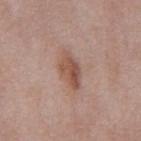Clinical impression:
This lesion was catalogued during total-body skin photography and was not selected for biopsy.
Acquisition and patient details:
Automated image analysis of the tile measured an outline eccentricity of about 0.85 (0 = round, 1 = elongated). It also reported an average lesion color of about L≈52 a*≈20 b*≈27 (CIELAB), roughly 10 lightness units darker than nearby skin, and a normalized border contrast of about 8. And it measured internal color variation of about 5.5 on a 0–10 scale and a peripheral color-asymmetry measure near 2. Captured under white-light illumination. The lesion is located on the chest. This image is a 15 mm lesion crop taken from a total-body photograph. A male patient approximately 55 years of age. Measured at roughly 4.5 mm in maximum diameter.